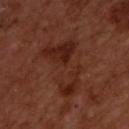Case summary:
• workup · no biopsy performed (imaged during a skin exam)
• patient · male, roughly 50 years of age
• body site · the upper back
• image · 15 mm crop, total-body photography